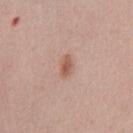<record>
  <biopsy_status>not biopsied; imaged during a skin examination</biopsy_status>
  <patient>
    <sex>female</sex>
    <age_approx>40</age_approx>
  </patient>
  <lighting>white-light</lighting>
  <lesion_size>
    <long_diameter_mm_approx>2.5</long_diameter_mm_approx>
  </lesion_size>
  <image>
    <source>total-body photography crop</source>
    <field_of_view_mm>15</field_of_view_mm>
  </image>
  <site>chest</site>
</record>